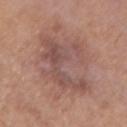Recorded during total-body skin imaging; not selected for excision or biopsy. A male subject aged approximately 75. The tile uses white-light illumination. The lesion's longest dimension is about 6 mm. On the left forearm. A roughly 15 mm field-of-view crop from a total-body skin photograph. The total-body-photography lesion software estimated a footprint of about 21 mm² and a symmetry-axis asymmetry near 0.55. The software also gave radial color variation of about 1.